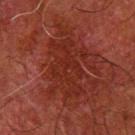Findings:
- biopsy status — total-body-photography surveillance lesion; no biopsy
- anatomic site — the right forearm
- image — ~15 mm crop, total-body skin-cancer survey
- patient — male, in their 80s